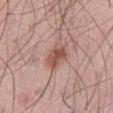The lesion was tiled from a total-body skin photograph and was not biopsied.
The lesion is located on the abdomen.
The tile uses white-light illumination.
A male subject aged 58–62.
This image is a 15 mm lesion crop taken from a total-body photograph.
The total-body-photography lesion software estimated a border-irregularity rating of about 3/10, a color-variation rating of about 3.5/10, and radial color variation of about 1. It also reported an automated nevus-likeness rating near 85 out of 100 and a lesion-detection confidence of about 100/100.